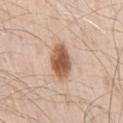The lesion was photographed on a routine skin check and not biopsied; there is no pathology result. Longest diameter approximately 4.5 mm. The patient is a male aged approximately 70. The lesion-visualizer software estimated an area of roughly 9.5 mm², an eccentricity of roughly 0.8, and a symmetry-axis asymmetry near 0.15. And it measured a mean CIELAB color near L≈57 a*≈21 b*≈32 and about 17 CIELAB-L* units darker than the surrounding skin. It also reported border irregularity of about 2 on a 0–10 scale and a peripheral color-asymmetry measure near 1. The software also gave a classifier nevus-likeness of about 100/100 and a lesion-detection confidence of about 100/100. A roughly 15 mm field-of-view crop from a total-body skin photograph. On the right upper arm.